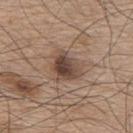Clinical impression: Imaged during a routine full-body skin examination; the lesion was not biopsied and no histopathology is available. Image and clinical context: From the upper back. Cropped from a whole-body photographic skin survey; the tile spans about 15 mm. Automated image analysis of the tile measured border irregularity of about 2.5 on a 0–10 scale. The subject is a male aged around 75.